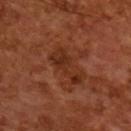Acquisition and patient details: Cropped from a whole-body photographic skin survey; the tile spans about 15 mm. The subject is a male aged around 65. Imaged with cross-polarized lighting.